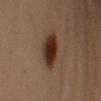This lesion was catalogued during total-body skin photography and was not selected for biopsy.
A male patient aged 53 to 57.
On the left upper arm.
Automated tile analysis of the lesion measured a lesion-to-skin contrast of about 12.5 (normalized; higher = more distinct). And it measured lesion-presence confidence of about 100/100.
Captured under cross-polarized illumination.
A roughly 15 mm field-of-view crop from a total-body skin photograph.